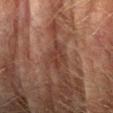Findings:
* follow-up — total-body-photography surveillance lesion; no biopsy
* body site — the arm
* patient — male, aged approximately 75
* lesion size — ≈3.5 mm
* lighting — cross-polarized illumination
* imaging modality — total-body-photography crop, ~15 mm field of view